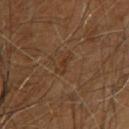Q: Lesion location?
A: the upper back
Q: What are the patient's age and sex?
A: female, in their mid- to late 60s
Q: Automated lesion metrics?
A: peripheral color asymmetry of about 0
Q: What is the lesion's diameter?
A: ~2.5 mm (longest diameter)
Q: How was this image acquired?
A: 15 mm crop, total-body photography